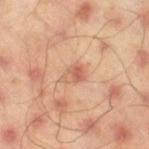Impression: This lesion was catalogued during total-body skin photography and was not selected for biopsy. Background: A 15 mm crop from a total-body photograph taken for skin-cancer surveillance. This is a cross-polarized tile. About 3 mm across. Automated tile analysis of the lesion measured a footprint of about 5 mm² and an eccentricity of roughly 0.7. It also reported an average lesion color of about L≈61 a*≈23 b*≈32 (CIELAB) and about 9 CIELAB-L* units darker than the surrounding skin. The subject is a male aged approximately 45. On the right thigh.